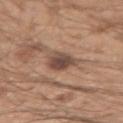Assessment: Captured during whole-body skin photography for melanoma surveillance; the lesion was not biopsied. Clinical summary: About 3.5 mm across. Located on the right upper arm. A roughly 15 mm field-of-view crop from a total-body skin photograph. A male patient, about 20 years old. The lesion-visualizer software estimated a shape eccentricity near 0.55 and a symmetry-axis asymmetry near 0.25. And it measured about 12 CIELAB-L* units darker than the surrounding skin and a normalized border contrast of about 9.5. The analysis additionally found a border-irregularity index near 2.5/10, a within-lesion color-variation index near 4.5/10, and a peripheral color-asymmetry measure near 1. It also reported a classifier nevus-likeness of about 15/100 and a detector confidence of about 100 out of 100 that the crop contains a lesion.